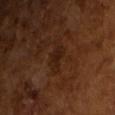biopsy status: imaged on a skin check; not biopsied | size: ~3 mm (longest diameter) | patient: male, in their mid- to late 60s | image source: ~15 mm tile from a whole-body skin photo | TBP lesion metrics: an area of roughly 4 mm², an outline eccentricity of about 0.8 (0 = round, 1 = elongated), and a shape-asymmetry score of about 0.55 (0 = symmetric); a mean CIELAB color near L≈19 a*≈18 b*≈24 and about 5 CIELAB-L* units darker than the surrounding skin | tile lighting: cross-polarized illumination.